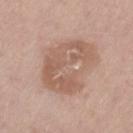notes — total-body-photography surveillance lesion; no biopsy | diameter — about 7 mm | patient — male, about 75 years old | lighting — white-light | anatomic site — the leg | image source — 15 mm crop, total-body photography.